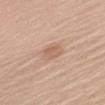Impression: Recorded during total-body skin imaging; not selected for excision or biopsy. Context: A female patient, aged approximately 40. The lesion is on the left upper arm. A 15 mm crop from a total-body photograph taken for skin-cancer surveillance. Imaged with white-light lighting. The total-body-photography lesion software estimated an area of roughly 4 mm², an outline eccentricity of about 0.8 (0 = round, 1 = elongated), and a shape-asymmetry score of about 0.15 (0 = symmetric). The analysis additionally found a lesion-detection confidence of about 100/100.